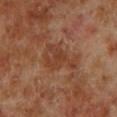Captured during whole-body skin photography for melanoma surveillance; the lesion was not biopsied.
Imaged with cross-polarized lighting.
The lesion-visualizer software estimated a lesion area of about 11 mm², a shape eccentricity near 0.8, and two-axis asymmetry of about 0.5. The software also gave a color-variation rating of about 3/10 and radial color variation of about 1. It also reported a detector confidence of about 100 out of 100 that the crop contains a lesion.
A 15 mm close-up tile from a total-body photography series done for melanoma screening.
The lesion's longest dimension is about 5 mm.
The patient is a male approximately 70 years of age.
On the left lower leg.